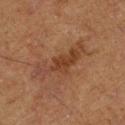This lesion was catalogued during total-body skin photography and was not selected for biopsy. A male patient, about 75 years old. Imaged with cross-polarized lighting. About 7 mm across. Located on the left lower leg. Cropped from a total-body skin-imaging series; the visible field is about 15 mm. Automated tile analysis of the lesion measured an average lesion color of about L≈32 a*≈18 b*≈26 (CIELAB), about 7 CIELAB-L* units darker than the surrounding skin, and a normalized lesion–skin contrast near 6.5. The analysis additionally found a border-irregularity index near 7/10, a color-variation rating of about 3.5/10, and radial color variation of about 1.